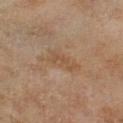No biopsy was performed on this lesion — it was imaged during a full skin examination and was not determined to be concerning. A 15 mm close-up tile from a total-body photography series done for melanoma screening. Automated tile analysis of the lesion measured a lesion area of about 6.5 mm², an eccentricity of roughly 0.9, and a symmetry-axis asymmetry near 0.4. It also reported a border-irregularity rating of about 4.5/10 and a within-lesion color-variation index near 2/10. The subject is a female approximately 60 years of age. On the leg. The recorded lesion diameter is about 4.5 mm. This is a cross-polarized tile.